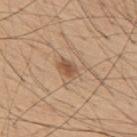Clinical summary: The total-body-photography lesion software estimated an area of roughly 4 mm², an outline eccentricity of about 0.8 (0 = round, 1 = elongated), and a shape-asymmetry score of about 0.2 (0 = symmetric). The analysis additionally found an average lesion color of about L≈53 a*≈19 b*≈32 (CIELAB), a lesion–skin lightness drop of about 11, and a normalized lesion–skin contrast near 7.5. It also reported a lesion-detection confidence of about 100/100. This is a white-light tile. The lesion is located on the upper back. A region of skin cropped from a whole-body photographic capture, roughly 15 mm wide. Approximately 3 mm at its widest. The subject is a male approximately 55 years of age.